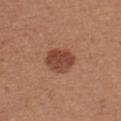| field | value |
|---|---|
| follow-up | catalogued during a skin exam; not biopsied |
| patient | female, roughly 25 years of age |
| automated metrics | a nevus-likeness score of about 90/100 and a detector confidence of about 100 out of 100 that the crop contains a lesion |
| imaging modality | ~15 mm tile from a whole-body skin photo |
| site | the chest |
| lesion size | ≈3.5 mm |